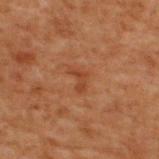{"biopsy_status": "not biopsied; imaged during a skin examination", "site": "upper back", "patient": {"sex": "male", "age_approx": 70}, "image": {"source": "total-body photography crop", "field_of_view_mm": 15}}